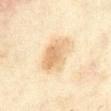Findings:
- notes: imaged on a skin check; not biopsied
- tile lighting: cross-polarized
- image source: ~15 mm tile from a whole-body skin photo
- automated lesion analysis: a mean CIELAB color near L≈73 a*≈15 b*≈38 and roughly 10 lightness units darker than nearby skin; a nevus-likeness score of about 65/100 and a lesion-detection confidence of about 100/100
- location: the abdomen
- subject: female, aged 33 to 37